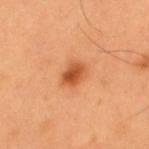Notes:
– follow-up · total-body-photography surveillance lesion; no biopsy
– subject · male, aged approximately 55
– TBP lesion metrics · an eccentricity of roughly 0.65; a border-irregularity index near 1.5/10 and internal color variation of about 4.5 on a 0–10 scale; an automated nevus-likeness rating near 95 out of 100
– lesion diameter · ≈3 mm
– anatomic site · the upper back
– acquisition · 15 mm crop, total-body photography